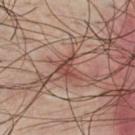Assessment:
No biopsy was performed on this lesion — it was imaged during a full skin examination and was not determined to be concerning.
Background:
The patient is a male aged around 35. The lesion is located on the chest. A roughly 15 mm field-of-view crop from a total-body skin photograph.